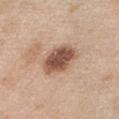workup: no biopsy performed (imaged during a skin exam)
patient: female, aged 38–42
image source: total-body-photography crop, ~15 mm field of view
size: about 4.5 mm
anatomic site: the chest
automated metrics: a lesion area of about 12 mm² and an outline eccentricity of about 0.75 (0 = round, 1 = elongated); a mean CIELAB color near L≈53 a*≈20 b*≈29, a lesion–skin lightness drop of about 16, and a normalized border contrast of about 10.5; a classifier nevus-likeness of about 90/100 and lesion-presence confidence of about 100/100